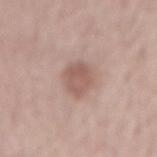Impression:
The lesion was tiled from a total-body skin photograph and was not biopsied.
Acquisition and patient details:
About 4 mm across. Cropped from a total-body skin-imaging series; the visible field is about 15 mm. Automated tile analysis of the lesion measured a footprint of about 8 mm² and a shape-asymmetry score of about 0.2 (0 = symmetric). The software also gave a border-irregularity rating of about 2/10, a within-lesion color-variation index near 2.5/10, and radial color variation of about 1. The software also gave a classifier nevus-likeness of about 15/100. This is a white-light tile. On the lower back. A male patient, aged 58 to 62.